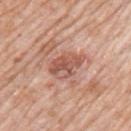Image and clinical context:
Automated image analysis of the tile measured an eccentricity of roughly 0.8 and a shape-asymmetry score of about 0.25 (0 = symmetric). The software also gave about 11 CIELAB-L* units darker than the surrounding skin and a normalized border contrast of about 7.5. And it measured a border-irregularity rating of about 3/10 and a color-variation rating of about 6/10. It also reported a lesion-detection confidence of about 100/100. A 15 mm close-up tile from a total-body photography series done for melanoma screening. A male subject, approximately 80 years of age. Located on the left upper arm. The tile uses white-light illumination.